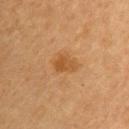Findings:
• workup — total-body-photography surveillance lesion; no biopsy
• image — ~15 mm tile from a whole-body skin photo
• body site — the right upper arm
• subject — female, about 60 years old
• lesion size — ~3 mm (longest diameter)